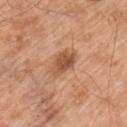Clinical impression: No biopsy was performed on this lesion — it was imaged during a full skin examination and was not determined to be concerning. Acquisition and patient details: A male subject, about 55 years old. The lesion is located on the arm. A region of skin cropped from a whole-body photographic capture, roughly 15 mm wide. Imaged with white-light lighting. The total-body-photography lesion software estimated an average lesion color of about L≈53 a*≈24 b*≈35 (CIELAB) and about 12 CIELAB-L* units darker than the surrounding skin. It also reported border irregularity of about 2 on a 0–10 scale, a within-lesion color-variation index near 4/10, and radial color variation of about 1.5. Approximately 3.5 mm at its widest.